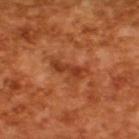Clinical impression: Recorded during total-body skin imaging; not selected for excision or biopsy. Image and clinical context: A close-up tile cropped from a whole-body skin photograph, about 15 mm across. A male subject in their mid- to late 60s.